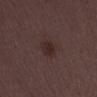Part of a total-body skin-imaging series; this lesion was reviewed on a skin check and was not flagged for biopsy. Imaged with white-light lighting. A male subject, aged approximately 50. An algorithmic analysis of the crop reported a lesion color around L≈26 a*≈16 b*≈16 in CIELAB, a lesion–skin lightness drop of about 6, and a lesion-to-skin contrast of about 7 (normalized; higher = more distinct). A close-up tile cropped from a whole-body skin photograph, about 15 mm across. Longest diameter approximately 3 mm.